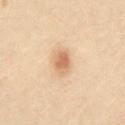Recorded during total-body skin imaging; not selected for excision or biopsy. The lesion is on the abdomen. A close-up tile cropped from a whole-body skin photograph, about 15 mm across. About 3 mm across. The patient is a female about 30 years old.